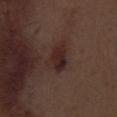  biopsy_status: not biopsied; imaged during a skin examination
  lighting: white-light
  patient:
    sex: male
    age_approx: 70
  automated_metrics:
    cielab_L: 25
    cielab_a: 17
    cielab_b: 18
    vs_skin_darker_L: 7.0
    vs_skin_contrast_norm: 8.0
  site: left thigh
  image:
    source: total-body photography crop
    field_of_view_mm: 15
  lesion_size:
    long_diameter_mm_approx: 4.0